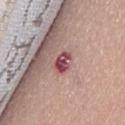Imaged during a routine full-body skin examination; the lesion was not biopsied and no histopathology is available. The lesion is located on the back. Captured under white-light illumination. The total-body-photography lesion software estimated a border-irregularity index near 2.5/10 and internal color variation of about 6.5 on a 0–10 scale. And it measured a lesion-detection confidence of about 100/100. A female subject, aged approximately 70. The recorded lesion diameter is about 3 mm. Cropped from a whole-body photographic skin survey; the tile spans about 15 mm.